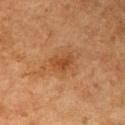Imaged during a routine full-body skin examination; the lesion was not biopsied and no histopathology is available. A female subject, aged 58 to 62. The lesion is located on the arm. A roughly 15 mm field-of-view crop from a total-body skin photograph. About 2.5 mm across. Imaged with cross-polarized lighting. Automated tile analysis of the lesion measured a footprint of about 4 mm² and a shape eccentricity near 0.75. It also reported a lesion color around L≈41 a*≈22 b*≈35 in CIELAB.